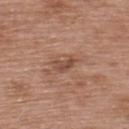This lesion was catalogued during total-body skin photography and was not selected for biopsy. On the upper back. A male patient, aged 48–52. Captured under white-light illumination. A 15 mm crop from a total-body photograph taken for skin-cancer surveillance. The lesion-visualizer software estimated a mean CIELAB color near L≈49 a*≈21 b*≈28, about 9 CIELAB-L* units darker than the surrounding skin, and a lesion-to-skin contrast of about 6.5 (normalized; higher = more distinct). The lesion's longest dimension is about 3 mm.